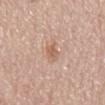  biopsy_status: not biopsied; imaged during a skin examination
  site: mid back
  patient:
    sex: male
    age_approx: 60
  image:
    source: total-body photography crop
    field_of_view_mm: 15
  lighting: white-light